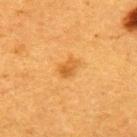lighting=cross-polarized illumination | imaging modality=15 mm crop, total-body photography | site=the upper back | patient=female, roughly 40 years of age | automated lesion analysis=a mean CIELAB color near L≈49 a*≈22 b*≈43 | size=≈2.5 mm.